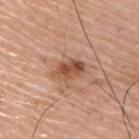Assessment:
Captured during whole-body skin photography for melanoma surveillance; the lesion was not biopsied.
Context:
A male patient, aged around 75. A 15 mm crop from a total-body photograph taken for skin-cancer surveillance. The lesion is on the upper back. Imaged with white-light lighting. Measured at roughly 4 mm in maximum diameter.